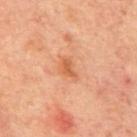imaging modality: 15 mm crop, total-body photography | anatomic site: the mid back | automated metrics: a shape eccentricity near 0.8 and a symmetry-axis asymmetry near 0.4; a mean CIELAB color near L≈58 a*≈28 b*≈39 and a lesion-to-skin contrast of about 7 (normalized; higher = more distinct); a detector confidence of about 100 out of 100 that the crop contains a lesion | size: ≈2.5 mm | subject: male, approximately 70 years of age | illumination: cross-polarized.